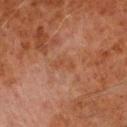<case>
<biopsy_status>not biopsied; imaged during a skin examination</biopsy_status>
<lesion_size>
  <long_diameter_mm_approx>3.0</long_diameter_mm_approx>
</lesion_size>
<site>right upper arm</site>
<image>
  <source>total-body photography crop</source>
  <field_of_view_mm>15</field_of_view_mm>
</image>
<patient>
  <sex>male</sex>
  <age_approx>70</age_approx>
</patient>
</case>